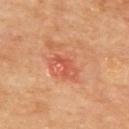Imaged during a routine full-body skin examination; the lesion was not biopsied and no histopathology is available. Captured under cross-polarized illumination. A roughly 15 mm field-of-view crop from a total-body skin photograph. Located on the upper back. A male subject, aged approximately 70. About 2.5 mm across.